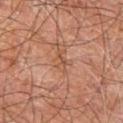biopsy status = catalogued during a skin exam; not biopsied | patient = male, aged approximately 60 | automated lesion analysis = border irregularity of about 4 on a 0–10 scale, a within-lesion color-variation index near 2.5/10, and radial color variation of about 1 | acquisition = ~15 mm tile from a whole-body skin photo | anatomic site = the right leg | tile lighting = cross-polarized illumination.